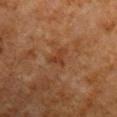biopsy_status: not biopsied; imaged during a skin examination
automated_metrics:
  cielab_L: 30
  cielab_a: 19
  cielab_b: 27
  vs_skin_darker_L: 6.0
  vs_skin_contrast_norm: 6.0
  nevus_likeness_0_100: 0
  lesion_detection_confidence_0_100: 100
lighting: cross-polarized
image:
  source: total-body photography crop
  field_of_view_mm: 15
site: arm
patient:
  sex: male
  age_approx: 80